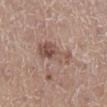Clinical impression:
The lesion was photographed on a routine skin check and not biopsied; there is no pathology result.
Context:
The subject is a female in their mid-40s. The lesion is on the right lower leg. A 15 mm close-up tile from a total-body photography series done for melanoma screening.